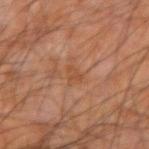Clinical impression:
Recorded during total-body skin imaging; not selected for excision or biopsy.
Image and clinical context:
The lesion is on the left forearm. The patient is a male in their mid-60s. Longest diameter approximately 2.5 mm. The tile uses cross-polarized illumination. The lesion-visualizer software estimated an area of roughly 3.5 mm² and an eccentricity of roughly 0.6. It also reported a border-irregularity rating of about 5.5/10, internal color variation of about 2 on a 0–10 scale, and a peripheral color-asymmetry measure near 1. A roughly 15 mm field-of-view crop from a total-body skin photograph.